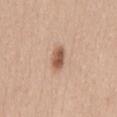workup: imaged on a skin check; not biopsied
site: the back
tile lighting: white-light illumination
subject: female, roughly 45 years of age
image: 15 mm crop, total-body photography
size: about 3 mm